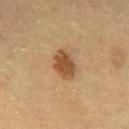{
  "biopsy_status": "not biopsied; imaged during a skin examination",
  "automated_metrics": {
    "area_mm2_approx": 7.5,
    "shape_asymmetry": 0.2
  },
  "patient": {
    "sex": "male",
    "age_approx": 60
  },
  "image": {
    "source": "total-body photography crop",
    "field_of_view_mm": 15
  },
  "site": "abdomen"
}